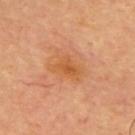workup: total-body-photography surveillance lesion; no biopsy
body site: the upper back
image: total-body-photography crop, ~15 mm field of view
image-analysis metrics: a shape eccentricity near 0.75 and a shape-asymmetry score of about 0.35 (0 = symmetric); an average lesion color of about L≈57 a*≈25 b*≈42 (CIELAB); a border-irregularity rating of about 4/10, a color-variation rating of about 3.5/10, and peripheral color asymmetry of about 1; a classifier nevus-likeness of about 0/100
subject: male, aged approximately 65